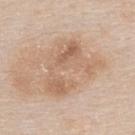No biopsy was performed on this lesion — it was imaged during a full skin examination and was not determined to be concerning. The lesion is located on the back. A close-up tile cropped from a whole-body skin photograph, about 15 mm across. The subject is a female aged 53 to 57. Measured at roughly 7 mm in maximum diameter. Imaged with white-light lighting.